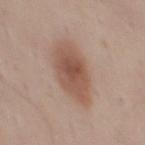follow-up — catalogued during a skin exam; not biopsied
lesion size — ≈6 mm
location — the mid back
image — ~15 mm tile from a whole-body skin photo
patient — male, approximately 35 years of age
tile lighting — white-light